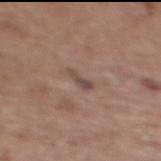follow-up = no biopsy performed (imaged during a skin exam) | location = the mid back | imaging modality = ~15 mm crop, total-body skin-cancer survey | size = about 3 mm | patient = female, in their mid-60s.